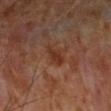Clinical impression: No biopsy was performed on this lesion — it was imaged during a full skin examination and was not determined to be concerning. Context: A lesion tile, about 15 mm wide, cut from a 3D total-body photograph. Captured under cross-polarized illumination. From the left forearm. A male patient roughly 70 years of age. About 3.5 mm across.